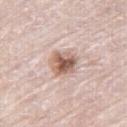Imaged during a routine full-body skin examination; the lesion was not biopsied and no histopathology is available. Cropped from a total-body skin-imaging series; the visible field is about 15 mm. Automated image analysis of the tile measured a mean CIELAB color near L≈60 a*≈19 b*≈26, roughly 15 lightness units darker than nearby skin, and a lesion-to-skin contrast of about 9.5 (normalized; higher = more distinct). It also reported a nevus-likeness score of about 85/100 and a lesion-detection confidence of about 100/100. The tile uses white-light illumination. The lesion is located on the right thigh. The lesion's longest dimension is about 3 mm. The subject is a male aged 78 to 82.